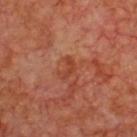Impression:
Imaged during a routine full-body skin examination; the lesion was not biopsied and no histopathology is available.
Context:
An algorithmic analysis of the crop reported a lesion area of about 3 mm², a shape eccentricity near 0.85, and a symmetry-axis asymmetry near 0.55. The software also gave a border-irregularity rating of about 5.5/10, internal color variation of about 0 on a 0–10 scale, and a peripheral color-asymmetry measure near 0. It also reported an automated nevus-likeness rating near 0 out of 100 and lesion-presence confidence of about 100/100. Approximately 2.5 mm at its widest. A lesion tile, about 15 mm wide, cut from a 3D total-body photograph. This is a cross-polarized tile. The lesion is on the chest. A male patient, roughly 65 years of age.